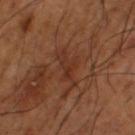Q: Was this lesion biopsied?
A: imaged on a skin check; not biopsied
Q: What lighting was used for the tile?
A: cross-polarized illumination
Q: Who is the patient?
A: male, aged approximately 65
Q: Where on the body is the lesion?
A: the left thigh
Q: What kind of image is this?
A: total-body-photography crop, ~15 mm field of view